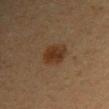Recorded during total-body skin imaging; not selected for excision or biopsy.